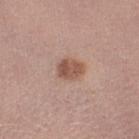Impression: Part of a total-body skin-imaging series; this lesion was reviewed on a skin check and was not flagged for biopsy. Image and clinical context: A male patient, aged approximately 30. Imaged with white-light lighting. About 3 mm across. A 15 mm close-up tile from a total-body photography series done for melanoma screening.